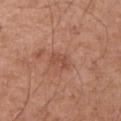biopsy status: imaged on a skin check; not biopsied
subject: male, aged approximately 55
acquisition: ~15 mm tile from a whole-body skin photo
site: the left upper arm
tile lighting: white-light illumination
diameter: ≈2.5 mm
automated metrics: an outline eccentricity of about 0.75 (0 = round, 1 = elongated); a lesion color around L≈50 a*≈24 b*≈29 in CIELAB, about 7 CIELAB-L* units darker than the surrounding skin, and a lesion-to-skin contrast of about 5 (normalized; higher = more distinct); border irregularity of about 5 on a 0–10 scale, a within-lesion color-variation index near 0.5/10, and radial color variation of about 0